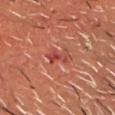Assessment:
Captured during whole-body skin photography for melanoma surveillance; the lesion was not biopsied.
Acquisition and patient details:
Cropped from a total-body skin-imaging series; the visible field is about 15 mm. The recorded lesion diameter is about 2.5 mm. Automated tile analysis of the lesion measured a classifier nevus-likeness of about 0/100 and lesion-presence confidence of about 95/100. A male patient, in their mid-60s. Imaged with cross-polarized lighting. From the head or neck.